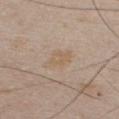Automated tile analysis of the lesion measured a footprint of about 7 mm², an outline eccentricity of about 0.8 (0 = round, 1 = elongated), and a symmetry-axis asymmetry near 0.2. The analysis additionally found a lesion-to-skin contrast of about 5 (normalized; higher = more distinct). The analysis additionally found a border-irregularity index near 3/10, internal color variation of about 1.5 on a 0–10 scale, and peripheral color asymmetry of about 0.5.
Captured under white-light illumination.
The lesion's longest dimension is about 4 mm.
On the chest.
A region of skin cropped from a whole-body photographic capture, roughly 15 mm wide.
The patient is a male approximately 45 years of age.